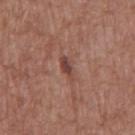{
  "biopsy_status": "not biopsied; imaged during a skin examination",
  "patient": {
    "sex": "male",
    "age_approx": 75
  },
  "image": {
    "source": "total-body photography crop",
    "field_of_view_mm": 15
  },
  "site": "abdomen",
  "automated_metrics": {
    "vs_skin_darker_L": 10.0,
    "vs_skin_contrast_norm": 8.0,
    "border_irregularity_0_10": 3.0,
    "peripheral_color_asymmetry": 0.0,
    "nevus_likeness_0_100": 70,
    "lesion_detection_confidence_0_100": 100
  }
}